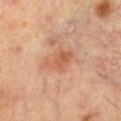No biopsy was performed on this lesion — it was imaged during a full skin examination and was not determined to be concerning. A female subject in their mid- to late 50s. A roughly 15 mm field-of-view crop from a total-body skin photograph. Located on the leg. Captured under cross-polarized illumination.